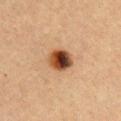Findings:
- notes · catalogued during a skin exam; not biopsied
- site · the chest
- lighting · cross-polarized
- lesion size · ≈3 mm
- patient · male, aged 38 to 42
- imaging modality · ~15 mm tile from a whole-body skin photo
- automated lesion analysis · a lesion color around L≈36 a*≈20 b*≈29 in CIELAB, about 18 CIELAB-L* units darker than the surrounding skin, and a normalized lesion–skin contrast near 14.5; a border-irregularity rating of about 1.5/10, a within-lesion color-variation index near 10/10, and peripheral color asymmetry of about 4